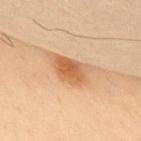<record>
<biopsy_status>not biopsied; imaged during a skin examination</biopsy_status>
<patient>
  <sex>male</sex>
  <age_approx>50</age_approx>
</patient>
<automated_metrics>
  <area_mm2_approx>11.0</area_mm2_approx>
  <eccentricity>0.85</eccentricity>
  <shape_asymmetry>0.25</shape_asymmetry>
  <cielab_L>61</cielab_L>
  <cielab_a>23</cielab_a>
  <cielab_b>39</cielab_b>
  <vs_skin_contrast_norm>8.0</vs_skin_contrast_norm>
</automated_metrics>
<site>upper back</site>
<image>
  <source>total-body photography crop</source>
  <field_of_view_mm>15</field_of_view_mm>
</image>
<lighting>cross-polarized</lighting>
<lesion_size>
  <long_diameter_mm_approx>5.0</long_diameter_mm_approx>
</lesion_size>
</record>